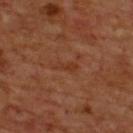This lesion was catalogued during total-body skin photography and was not selected for biopsy. Located on the upper back. Imaged with cross-polarized lighting. A 15 mm close-up extracted from a 3D total-body photography capture. Approximately 3 mm at its widest. The patient is a male in their 70s.